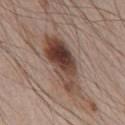Q: Was this lesion biopsied?
A: imaged on a skin check; not biopsied
Q: How was this image acquired?
A: 15 mm crop, total-body photography
Q: What is the anatomic site?
A: the chest
Q: Who is the patient?
A: male, aged 63–67
Q: Illumination type?
A: white-light
Q: How large is the lesion?
A: about 8.5 mm